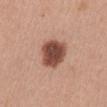<record>
<biopsy_status>not biopsied; imaged during a skin examination</biopsy_status>
<image>
  <source>total-body photography crop</source>
  <field_of_view_mm>15</field_of_view_mm>
</image>
<site>front of the torso</site>
<patient>
  <sex>female</sex>
  <age_approx>40</age_approx>
</patient>
<lighting>white-light</lighting>
<automated_metrics>
  <shape_asymmetry>0.15</shape_asymmetry>
  <cielab_L>48</cielab_L>
  <cielab_a>23</cielab_a>
  <cielab_b>27</cielab_b>
  <vs_skin_darker_L>17.0</vs_skin_darker_L>
  <vs_skin_contrast_norm>12.0</vs_skin_contrast_norm>
  <nevus_likeness_0_100>75</nevus_likeness_0_100>
  <lesion_detection_confidence_0_100>100</lesion_detection_confidence_0_100>
</automated_metrics>
</record>